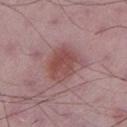Measured at roughly 4.5 mm in maximum diameter. A 15 mm close-up extracted from a 3D total-body photography capture. Captured under white-light illumination. The patient is a male approximately 50 years of age. On the left lower leg.